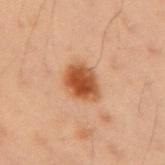Context:
A female subject, in their 40s. Captured under cross-polarized illumination. A roughly 15 mm field-of-view crop from a total-body skin photograph. About 4 mm across. The lesion is on the right upper arm.